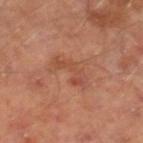<tbp_lesion>
  <biopsy_status>not biopsied; imaged during a skin examination</biopsy_status>
  <image>
    <source>total-body photography crop</source>
    <field_of_view_mm>15</field_of_view_mm>
  </image>
  <patient>
    <sex>male</sex>
    <age_approx>65</age_approx>
  </patient>
  <site>right lower leg</site>
</tbp_lesion>